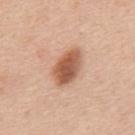biopsy_status: not biopsied; imaged during a skin examination
lesion_size:
  long_diameter_mm_approx: 4.5
automated_metrics:
  area_mm2_approx: 10.0
  eccentricity: 0.8
  shape_asymmetry: 0.15
  color_variation_0_10: 4.5
  peripheral_color_asymmetry: 1.5
  lesion_detection_confidence_0_100: 100
site: mid back
patient:
  sex: male
  age_approx: 50
lighting: white-light
image:
  source: total-body photography crop
  field_of_view_mm: 15